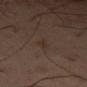No biopsy was performed on this lesion — it was imaged during a full skin examination and was not determined to be concerning.
On the left thigh.
Cropped from a whole-body photographic skin survey; the tile spans about 15 mm.
An algorithmic analysis of the crop reported a footprint of about 2.5 mm² and a symmetry-axis asymmetry near 0.3. The analysis additionally found a mean CIELAB color near L≈30 a*≈13 b*≈20 and a normalized lesion–skin contrast near 5. And it measured a nevus-likeness score of about 0/100.
The lesion's longest dimension is about 3 mm.
The patient is a male in their mid- to late 50s.
Imaged with cross-polarized lighting.